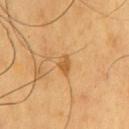<lesion>
<biopsy_status>not biopsied; imaged during a skin examination</biopsy_status>
<image>
  <source>total-body photography crop</source>
  <field_of_view_mm>15</field_of_view_mm>
</image>
<automated_metrics>
  <area_mm2_approx>3.0</area_mm2_approx>
  <shape_asymmetry>0.35</shape_asymmetry>
  <nevus_likeness_0_100>30</nevus_likeness_0_100>
  <lesion_detection_confidence_0_100>100</lesion_detection_confidence_0_100>
</automated_metrics>
<site>chest</site>
<patient>
  <sex>male</sex>
  <age_approx>60</age_approx>
</patient>
<lighting>cross-polarized</lighting>
<lesion_size>
  <long_diameter_mm_approx>2.5</long_diameter_mm_approx>
</lesion_size>
</lesion>